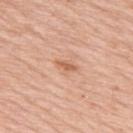  biopsy_status: not biopsied; imaged during a skin examination
  image:
    source: total-body photography crop
    field_of_view_mm: 15
  site: upper back
  patient:
    sex: male
    age_approx: 80
  lighting: white-light